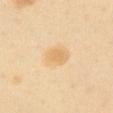Impression:
Captured during whole-body skin photography for melanoma surveillance; the lesion was not biopsied.
Context:
A 15 mm close-up tile from a total-body photography series done for melanoma screening. Located on the abdomen. A female patient, in their 40s. This is a cross-polarized tile. The total-body-photography lesion software estimated a shape eccentricity near 0.45 and a shape-asymmetry score of about 0.15 (0 = symmetric). The software also gave a lesion color around L≈76 a*≈15 b*≈44 in CIELAB, a lesion–skin lightness drop of about 7, and a normalized border contrast of about 6. And it measured a within-lesion color-variation index near 2.5/10 and peripheral color asymmetry of about 1.